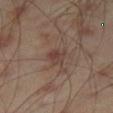  biopsy_status: not biopsied; imaged during a skin examination
  patient:
    sex: male
    age_approx: 45
  image:
    source: total-body photography crop
    field_of_view_mm: 15
  site: right thigh
  lighting: cross-polarized
  automated_metrics:
    border_irregularity_0_10: 2.0
    color_variation_0_10: 2.5
    peripheral_color_asymmetry: 1.0
  lesion_size:
    long_diameter_mm_approx: 2.5